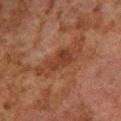Assessment:
The lesion was tiled from a total-body skin photograph and was not biopsied.
Clinical summary:
The lesion is on the right lower leg. A male subject aged approximately 80. A 15 mm close-up extracted from a 3D total-body photography capture.